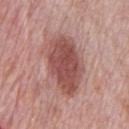The lesion was photographed on a routine skin check and not biopsied; there is no pathology result. About 7.5 mm across. A lesion tile, about 15 mm wide, cut from a 3D total-body photograph. The lesion is on the chest. A male subject, about 70 years old.